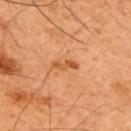This lesion was catalogued during total-body skin photography and was not selected for biopsy. From the upper back. A male patient, aged around 65. A 15 mm close-up tile from a total-body photography series done for melanoma screening.